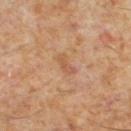<case>
  <biopsy_status>not biopsied; imaged during a skin examination</biopsy_status>
  <image>
    <source>total-body photography crop</source>
    <field_of_view_mm>15</field_of_view_mm>
  </image>
  <patient>
    <sex>male</sex>
    <age_approx>60</age_approx>
  </patient>
  <lesion_size>
    <long_diameter_mm_approx>2.5</long_diameter_mm_approx>
  </lesion_size>
  <lighting>cross-polarized</lighting>
  <site>left lower leg</site>
</case>